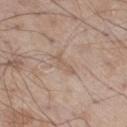The lesion was photographed on a routine skin check and not biopsied; there is no pathology result. A 15 mm crop from a total-body photograph taken for skin-cancer surveillance. This is a white-light tile. Measured at roughly 3.5 mm in maximum diameter. The lesion is located on the leg. A male subject roughly 55 years of age.